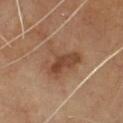follow-up = imaged on a skin check; not biopsied.